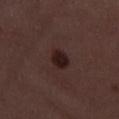Q: What kind of image is this?
A: total-body-photography crop, ~15 mm field of view
Q: Where on the body is the lesion?
A: the left thigh
Q: Patient demographics?
A: female, aged around 50
Q: How was the tile lit?
A: white-light